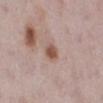Q: What kind of image is this?
A: total-body-photography crop, ~15 mm field of view
Q: Lesion location?
A: the right lower leg
Q: Patient demographics?
A: female, aged 28 to 32